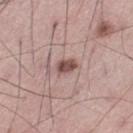Imaged during a routine full-body skin examination; the lesion was not biopsied and no histopathology is available. The lesion is on the left thigh. Imaged with white-light lighting. Longest diameter approximately 2.5 mm. Cropped from a whole-body photographic skin survey; the tile spans about 15 mm. The patient is a male aged 58 to 62.